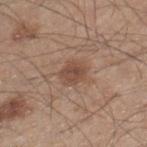{
  "biopsy_status": "not biopsied; imaged during a skin examination",
  "automated_metrics": {
    "area_mm2_approx": 5.5,
    "eccentricity": 0.55,
    "shape_asymmetry": 0.25,
    "cielab_L": 47,
    "cielab_a": 19,
    "cielab_b": 27,
    "vs_skin_darker_L": 9.0,
    "vs_skin_contrast_norm": 7.0
  },
  "image": {
    "source": "total-body photography crop",
    "field_of_view_mm": 15
  },
  "site": "leg",
  "lighting": "white-light",
  "patient": {
    "sex": "male",
    "age_approx": 45
  },
  "lesion_size": {
    "long_diameter_mm_approx": 3.0
  }
}